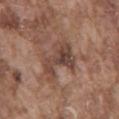Clinical summary: The lesion is located on the mid back. A male patient, in their mid-70s. A 15 mm close-up tile from a total-body photography series done for melanoma screening.